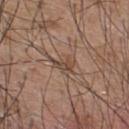biopsy_status: not biopsied; imaged during a skin examination
lesion_size:
  long_diameter_mm_approx: 3.0
automated_metrics:
  eccentricity: 0.8
  shape_asymmetry: 0.5
  vs_skin_darker_L: 9.0
  vs_skin_contrast_norm: 7.0
  border_irregularity_0_10: 6.0
  peripheral_color_asymmetry: 1.0
patient:
  sex: male
  age_approx: 55
site: upper back
lighting: white-light
image:
  source: total-body photography crop
  field_of_view_mm: 15